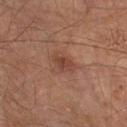notes — imaged on a skin check; not biopsied | subject — male, approximately 65 years of age | body site — the right lower leg | lesion diameter — about 3 mm | acquisition — 15 mm crop, total-body photography.